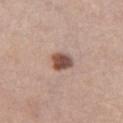Impression:
Part of a total-body skin-imaging series; this lesion was reviewed on a skin check and was not flagged for biopsy.
Context:
The lesion-visualizer software estimated a border-irregularity index near 1.5/10 and a within-lesion color-variation index near 3.5/10. The patient is a female aged approximately 65. The recorded lesion diameter is about 2.5 mm. Located on the chest. This image is a 15 mm lesion crop taken from a total-body photograph. This is a white-light tile.